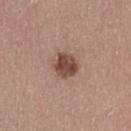| field | value |
|---|---|
| workup | catalogued during a skin exam; not biopsied |
| anatomic site | the leg |
| patient | female, aged approximately 45 |
| automated metrics | an average lesion color of about L≈46 a*≈21 b*≈24 (CIELAB), roughly 14 lightness units darker than nearby skin, and a normalized border contrast of about 10; a border-irregularity rating of about 2/10 and internal color variation of about 4.5 on a 0–10 scale; a nevus-likeness score of about 85/100 and a lesion-detection confidence of about 100/100 |
| imaging modality | ~15 mm crop, total-body skin-cancer survey |